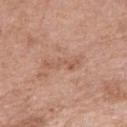{"biopsy_status": "not biopsied; imaged during a skin examination", "image": {"source": "total-body photography crop", "field_of_view_mm": 15}, "site": "right upper arm", "patient": {"sex": "female", "age_approx": 70}, "lighting": "white-light", "lesion_size": {"long_diameter_mm_approx": 4.5}, "automated_metrics": {"cielab_L": 57, "cielab_a": 21, "cielab_b": 30, "vs_skin_contrast_norm": 5.0}}